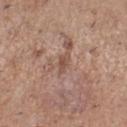Findings:
* biopsy status: total-body-photography surveillance lesion; no biopsy
* lesion size: about 2.5 mm
* subject: female, in their 30s
* illumination: white-light illumination
* anatomic site: the right lower leg
* image: 15 mm crop, total-body photography